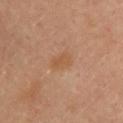The lesion was tiled from a total-body skin photograph and was not biopsied. The lesion is on the upper back. Approximately 3 mm at its widest. A roughly 15 mm field-of-view crop from a total-body skin photograph. The tile uses cross-polarized illumination. A female subject, about 35 years old.